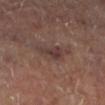Clinical impression:
The lesion was photographed on a routine skin check and not biopsied; there is no pathology result.
Image and clinical context:
Captured under cross-polarized illumination. The lesion is located on the left lower leg. A lesion tile, about 15 mm wide, cut from a 3D total-body photograph. An algorithmic analysis of the crop reported a nevus-likeness score of about 0/100. Approximately 4 mm at its widest.